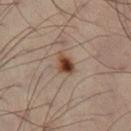- biopsy status · total-body-photography surveillance lesion; no biopsy
- lighting · cross-polarized illumination
- image-analysis metrics · a mean CIELAB color near L≈42 a*≈18 b*≈28, a lesion–skin lightness drop of about 14, and a normalized border contrast of about 11.5
- size · about 3 mm
- patient · male, aged around 50
- anatomic site · the left leg
- image · total-body-photography crop, ~15 mm field of view